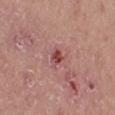Captured during whole-body skin photography for melanoma surveillance; the lesion was not biopsied. A female patient, roughly 40 years of age. A 15 mm close-up tile from a total-body photography series done for melanoma screening. The recorded lesion diameter is about 2.5 mm. From the right thigh. The tile uses white-light illumination. An algorithmic analysis of the crop reported two-axis asymmetry of about 0.3.